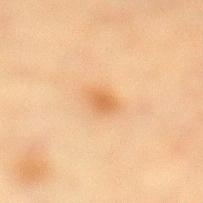Assessment: The lesion was tiled from a total-body skin photograph and was not biopsied. Image and clinical context: A female subject aged around 55. The lesion's longest dimension is about 3 mm. A lesion tile, about 15 mm wide, cut from a 3D total-body photograph. Located on the left lower leg.